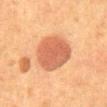{
  "patient": {
    "sex": "female",
    "age_approx": 50
  },
  "automated_metrics": {
    "eccentricity": 0.4,
    "shape_asymmetry": 0.1,
    "cielab_L": 50,
    "cielab_a": 24,
    "cielab_b": 32,
    "vs_skin_darker_L": 11.0,
    "vs_skin_contrast_norm": 8.0,
    "peripheral_color_asymmetry": 1.0,
    "nevus_likeness_0_100": 100,
    "lesion_detection_confidence_0_100": 100
  },
  "image": {
    "source": "total-body photography crop",
    "field_of_view_mm": 15
  },
  "lighting": "cross-polarized",
  "site": "abdomen",
  "lesion_size": {
    "long_diameter_mm_approx": 5.0
  }
}